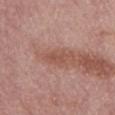This lesion was catalogued during total-body skin photography and was not selected for biopsy. A lesion tile, about 15 mm wide, cut from a 3D total-body photograph. Located on the abdomen. This is a white-light tile. A male subject, in their mid-70s. About 3 mm across.